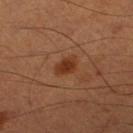Clinical impression:
No biopsy was performed on this lesion — it was imaged during a full skin examination and was not determined to be concerning.
Image and clinical context:
The total-body-photography lesion software estimated an outline eccentricity of about 0.75 (0 = round, 1 = elongated) and a shape-asymmetry score of about 0.25 (0 = symmetric). It also reported border irregularity of about 2 on a 0–10 scale and a color-variation rating of about 1.5/10. It also reported a lesion-detection confidence of about 100/100. A male subject in their 50s. A 15 mm crop from a total-body photograph taken for skin-cancer surveillance. The lesion is on the right thigh. The lesion's longest dimension is about 2.5 mm.